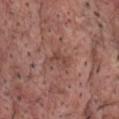| key | value |
|---|---|
| follow-up | total-body-photography surveillance lesion; no biopsy |
| illumination | white-light illumination |
| acquisition | ~15 mm tile from a whole-body skin photo |
| automated lesion analysis | a mean CIELAB color near L≈44 a*≈22 b*≈25, about 7 CIELAB-L* units darker than the surrounding skin, and a normalized border contrast of about 5.5; a lesion-detection confidence of about 100/100 |
| anatomic site | the chest |
| subject | male, in their 60s |
| diameter | ~2.5 mm (longest diameter) |